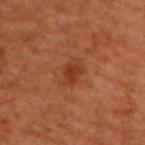Assessment: No biopsy was performed on this lesion — it was imaged during a full skin examination and was not determined to be concerning. Acquisition and patient details: Measured at roughly 3.5 mm in maximum diameter. The total-body-photography lesion software estimated an area of roughly 6 mm², an eccentricity of roughly 0.7, and a shape-asymmetry score of about 0.25 (0 = symmetric). It also reported an average lesion color of about L≈39 a*≈28 b*≈35 (CIELAB), about 8 CIELAB-L* units darker than the surrounding skin, and a normalized border contrast of about 6.5. On the upper back. A male patient, aged 48 to 52. Imaged with cross-polarized lighting. Cropped from a whole-body photographic skin survey; the tile spans about 15 mm.